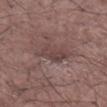<tbp_lesion>
<biopsy_status>not biopsied; imaged during a skin examination</biopsy_status>
<lesion_size>
  <long_diameter_mm_approx>4.0</long_diameter_mm_approx>
</lesion_size>
<site>leg</site>
<image>
  <source>total-body photography crop</source>
  <field_of_view_mm>15</field_of_view_mm>
</image>
<lighting>white-light</lighting>
<patient>
  <sex>male</sex>
  <age_approx>70</age_approx>
</patient>
</tbp_lesion>